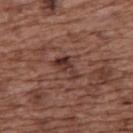{"biopsy_status": "not biopsied; imaged during a skin examination", "image": {"source": "total-body photography crop", "field_of_view_mm": 15}, "lighting": "white-light", "patient": {"sex": "female", "age_approx": 75}, "site": "upper back", "lesion_size": {"long_diameter_mm_approx": 3.5}}